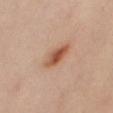Image and clinical context: A region of skin cropped from a whole-body photographic capture, roughly 15 mm wide. The tile uses cross-polarized illumination. The lesion is located on the left leg. Automated image analysis of the tile measured a lesion area of about 7 mm² and an outline eccentricity of about 0.9 (0 = round, 1 = elongated). And it measured roughly 12 lightness units darker than nearby skin and a normalized border contrast of about 8.5. The analysis additionally found a border-irregularity rating of about 2.5/10, a color-variation rating of about 7/10, and peripheral color asymmetry of about 2.5. The subject is a female in their 40s.